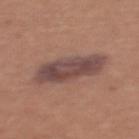The lesion was photographed on a routine skin check and not biopsied; there is no pathology result.
On the front of the torso.
The tile uses white-light illumination.
This image is a 15 mm lesion crop taken from a total-body photograph.
Approximately 6.5 mm at its widest.
Automated image analysis of the tile measured a lesion area of about 18 mm², an outline eccentricity of about 0.85 (0 = round, 1 = elongated), and a shape-asymmetry score of about 0.2 (0 = symmetric). And it measured a mean CIELAB color near L≈46 a*≈19 b*≈21, a lesion–skin lightness drop of about 11, and a lesion-to-skin contrast of about 9.5 (normalized; higher = more distinct). The analysis additionally found border irregularity of about 2.5 on a 0–10 scale, internal color variation of about 4 on a 0–10 scale, and peripheral color asymmetry of about 1.5.
The patient is a female aged 33–37.